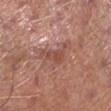Case summary:
* notes: total-body-photography surveillance lesion; no biopsy
* size: about 5 mm
* patient: male, in their mid- to late 60s
* lighting: white-light
* body site: the left lower leg
* automated metrics: a footprint of about 8.5 mm², an eccentricity of roughly 0.85, and a shape-asymmetry score of about 0.6 (0 = symmetric); an average lesion color of about L≈50 a*≈24 b*≈26 (CIELAB) and a lesion-to-skin contrast of about 6 (normalized; higher = more distinct); a border-irregularity index near 7.5/10, a within-lesion color-variation index near 2.5/10, and radial color variation of about 0.5
* image source: ~15 mm crop, total-body skin-cancer survey